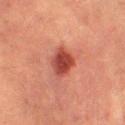Recorded during total-body skin imaging; not selected for excision or biopsy. The lesion is on the left lower leg. Measured at roughly 3.5 mm in maximum diameter. A female subject, roughly 55 years of age. Automated tile analysis of the lesion measured a mean CIELAB color near L≈37 a*≈28 b*≈26 and roughly 12 lightness units darker than nearby skin. And it measured a border-irregularity index near 2/10, a color-variation rating of about 3/10, and peripheral color asymmetry of about 1. The analysis additionally found a classifier nevus-likeness of about 95/100 and a lesion-detection confidence of about 100/100. Cropped from a total-body skin-imaging series; the visible field is about 15 mm. This is a cross-polarized tile.